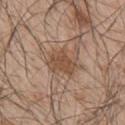This lesion was catalogued during total-body skin photography and was not selected for biopsy. The lesion-visualizer software estimated a lesion area of about 9 mm². It also reported a border-irregularity rating of about 3.5/10, internal color variation of about 2.5 on a 0–10 scale, and peripheral color asymmetry of about 1. And it measured a lesion-detection confidence of about 100/100. The recorded lesion diameter is about 4 mm. Located on the right upper arm. A lesion tile, about 15 mm wide, cut from a 3D total-body photograph. A male patient in their 50s.